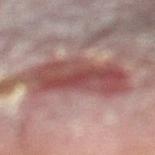Recorded during total-body skin imaging; not selected for excision or biopsy. A roughly 15 mm field-of-view crop from a total-body skin photograph. The lesion-visualizer software estimated a footprint of about 29 mm², an eccentricity of roughly 0.9, and a shape-asymmetry score of about 0.25 (0 = symmetric). And it measured a lesion color around L≈42 a*≈22 b*≈19 in CIELAB and a lesion-to-skin contrast of about 9 (normalized; higher = more distinct). It also reported border irregularity of about 3.5 on a 0–10 scale, internal color variation of about 6 on a 0–10 scale, and peripheral color asymmetry of about 2. The lesion is located on the left lower leg. The patient is a male aged 53–57. Imaged with cross-polarized lighting. About 9.5 mm across.